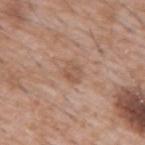Impression:
The lesion was photographed on a routine skin check and not biopsied; there is no pathology result.
Acquisition and patient details:
A male patient about 60 years old. Longest diameter approximately 2.5 mm. The lesion is on the chest. The tile uses white-light illumination. A roughly 15 mm field-of-view crop from a total-body skin photograph.